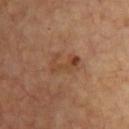Image and clinical context:
Longest diameter approximately 4 mm. From the chest. This image is a 15 mm lesion crop taken from a total-body photograph. The patient is aged 63–67. The tile uses cross-polarized illumination.